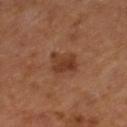Captured during whole-body skin photography for melanoma surveillance; the lesion was not biopsied. A roughly 15 mm field-of-view crop from a total-body skin photograph. The lesion-visualizer software estimated a mean CIELAB color near L≈36 a*≈23 b*≈30, about 8 CIELAB-L* units darker than the surrounding skin, and a normalized lesion–skin contrast near 7.5. It also reported a border-irregularity index near 3/10, internal color variation of about 3.5 on a 0–10 scale, and peripheral color asymmetry of about 1. Measured at roughly 3 mm in maximum diameter. This is a cross-polarized tile. From the leg. A female subject, aged 63 to 67.